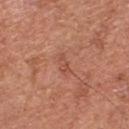follow-up: no biopsy performed (imaged during a skin exam)
illumination: white-light
acquisition: total-body-photography crop, ~15 mm field of view
anatomic site: the front of the torso
patient: male, aged around 65
automated metrics: a footprint of about 3 mm², a shape eccentricity near 0.9, and a shape-asymmetry score of about 0.25 (0 = symmetric); about 7 CIELAB-L* units darker than the surrounding skin and a normalized lesion–skin contrast near 5; a border-irregularity rating of about 3/10, a within-lesion color-variation index near 0.5/10, and peripheral color asymmetry of about 0